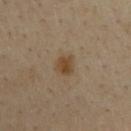Impression:
The lesion was photographed on a routine skin check and not biopsied; there is no pathology result.
Clinical summary:
A male patient in their mid-60s. On the chest. Cropped from a total-body skin-imaging series; the visible field is about 15 mm.